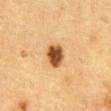Q: Was this lesion biopsied?
A: imaged on a skin check; not biopsied
Q: Patient demographics?
A: male, aged 83 or over
Q: Lesion size?
A: about 3 mm
Q: How was the tile lit?
A: cross-polarized
Q: Lesion location?
A: the abdomen
Q: What kind of image is this?
A: total-body-photography crop, ~15 mm field of view